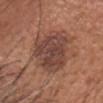Notes:
* biopsy status: total-body-photography surveillance lesion; no biopsy
* patient: male, aged approximately 60
* lighting: white-light
* image: 15 mm crop, total-body photography
* diameter: ≈6.5 mm
* location: the head or neck